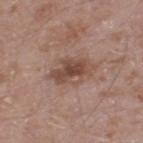{"biopsy_status": "not biopsied; imaged during a skin examination", "lesion_size": {"long_diameter_mm_approx": 5.0}, "image": {"source": "total-body photography crop", "field_of_view_mm": 15}, "lighting": "white-light", "site": "right thigh", "patient": {"sex": "male", "age_approx": 60}}